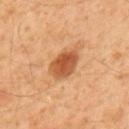biopsy_status: not biopsied; imaged during a skin examination
image:
  source: total-body photography crop
  field_of_view_mm: 15
lighting: cross-polarized
patient:
  sex: male
  age_approx: 60
site: upper back
automated_metrics:
  area_mm2_approx: 9.0
  shape_asymmetry: 0.2
  cielab_L: 50
  cielab_a: 25
  cielab_b: 37
  vs_skin_darker_L: 13.0
  vs_skin_contrast_norm: 9.0
  border_irregularity_0_10: 2.0
  color_variation_0_10: 4.0
  peripheral_color_asymmetry: 1.0
  nevus_likeness_0_100: 95
lesion_size:
  long_diameter_mm_approx: 4.5